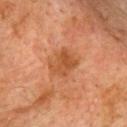Clinical impression: No biopsy was performed on this lesion — it was imaged during a full skin examination and was not determined to be concerning. Acquisition and patient details: Automated image analysis of the tile measured an area of roughly 8 mm² and a shape-asymmetry score of about 0.25 (0 = symmetric). The software also gave a border-irregularity rating of about 2.5/10 and a within-lesion color-variation index near 3.5/10. Imaged with cross-polarized lighting. Located on the upper back. Cropped from a total-body skin-imaging series; the visible field is about 15 mm. The subject is a male approximately 75 years of age.